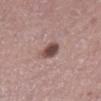This lesion was catalogued during total-body skin photography and was not selected for biopsy.
A male patient about 45 years old.
A 15 mm close-up tile from a total-body photography series done for melanoma screening.
On the left lower leg.
The tile uses white-light illumination.
Measured at roughly 3 mm in maximum diameter.